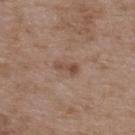<tbp_lesion>
  <biopsy_status>not biopsied; imaged during a skin examination</biopsy_status>
  <image>
    <source>total-body photography crop</source>
    <field_of_view_mm>15</field_of_view_mm>
  </image>
  <site>upper back</site>
  <lighting>white-light</lighting>
  <automated_metrics>
    <cielab_L>48</cielab_L>
    <cielab_a>18</cielab_a>
    <cielab_b>28</cielab_b>
    <vs_skin_darker_L>9.0</vs_skin_darker_L>
    <vs_skin_contrast_norm>7.0</vs_skin_contrast_norm>
  </automated_metrics>
  <lesion_size>
    <long_diameter_mm_approx>3.0</long_diameter_mm_approx>
  </lesion_size>
  <patient>
    <sex>male</sex>
    <age_approx>50</age_approx>
  </patient>
</tbp_lesion>